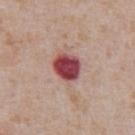Q: Is there a histopathology result?
A: no biopsy performed (imaged during a skin exam)
Q: Patient demographics?
A: male, aged 63–67
Q: What lighting was used for the tile?
A: white-light
Q: How was this image acquired?
A: total-body-photography crop, ~15 mm field of view
Q: Lesion location?
A: the abdomen